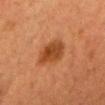  biopsy_status: not biopsied; imaged during a skin examination
  lighting: cross-polarized
  patient:
    sex: female
    age_approx: 40
  site: head or neck
  image:
    source: total-body photography crop
    field_of_view_mm: 15
  lesion_size:
    long_diameter_mm_approx: 4.5